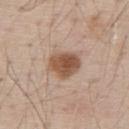Case summary:
- workup — catalogued during a skin exam; not biopsied
- lighting — white-light
- location — the upper back
- imaging modality — ~15 mm tile from a whole-body skin photo
- patient — male, aged 68 to 72